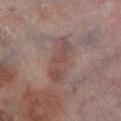{
  "patient": {
    "sex": "male",
    "age_approx": 65
  },
  "automated_metrics": {
    "eccentricity": 0.95,
    "shape_asymmetry": 0.3,
    "vs_skin_darker_L": 7.0,
    "vs_skin_contrast_norm": 6.0,
    "border_irregularity_0_10": 4.0,
    "color_variation_0_10": 3.5,
    "lesion_detection_confidence_0_100": 90
  },
  "lesion_size": {
    "long_diameter_mm_approx": 6.0
  },
  "image": {
    "source": "total-body photography crop",
    "field_of_view_mm": 15
  },
  "lighting": "cross-polarized",
  "site": "left lower leg"
}